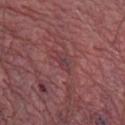Background:
Imaged with white-light lighting. About 2.5 mm across. The patient is a male aged 38–42. The lesion is located on the abdomen. A lesion tile, about 15 mm wide, cut from a 3D total-body photograph.